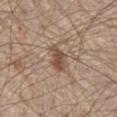biopsy_status: not biopsied; imaged during a skin examination
image:
  source: total-body photography crop
  field_of_view_mm: 15
site: abdomen
patient:
  sex: male
  age_approx: 80
automated_metrics:
  eccentricity: 0.9
  shape_asymmetry: 0.25
  vs_skin_darker_L: 11.0
  vs_skin_contrast_norm: 8.5
  color_variation_0_10: 3.0
  peripheral_color_asymmetry: 1.0
lighting: white-light
lesion_size:
  long_diameter_mm_approx: 3.5